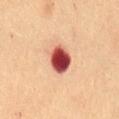<record>
  <patient>
    <sex>female</sex>
    <age_approx>70</age_approx>
  </patient>
  <image>
    <source>total-body photography crop</source>
    <field_of_view_mm>15</field_of_view_mm>
  </image>
  <lighting>cross-polarized</lighting>
  <site>back</site>
  <automated_metrics>
    <area_mm2_approx>8.5</area_mm2_approx>
    <eccentricity>0.65</eccentricity>
    <shape_asymmetry>0.15</shape_asymmetry>
    <cielab_L>44</cielab_L>
    <cielab_a>31</cielab_a>
    <cielab_b>26</cielab_b>
    <vs_skin_contrast_norm>15.0</vs_skin_contrast_norm>
    <nevus_likeness_0_100>0</nevus_likeness_0_100>
    <lesion_detection_confidence_0_100>100</lesion_detection_confidence_0_100>
  </automated_metrics>
</record>